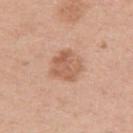The lesion was tiled from a total-body skin photograph and was not biopsied. On the left upper arm. Cropped from a total-body skin-imaging series; the visible field is about 15 mm. The recorded lesion diameter is about 4 mm. Captured under white-light illumination. The subject is a female aged around 40.